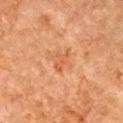{
  "biopsy_status": "not biopsied; imaged during a skin examination",
  "image": {
    "source": "total-body photography crop",
    "field_of_view_mm": 15
  },
  "site": "chest",
  "lighting": "cross-polarized",
  "patient": {
    "sex": "male",
    "age_approx": 80
  }
}